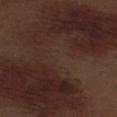notes — catalogued during a skin exam; not biopsied
diameter — ~17.5 mm (longest diameter)
TBP lesion metrics — a footprint of about 145 mm², an outline eccentricity of about 0.75 (0 = round, 1 = elongated), and a symmetry-axis asymmetry near 0.35; lesion-presence confidence of about 100/100
illumination — white-light illumination
acquisition — ~15 mm crop, total-body skin-cancer survey
location — the left thigh
patient — male, about 70 years old